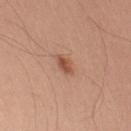A roughly 15 mm field-of-view crop from a total-body skin photograph.
From the arm.
A male subject, in their mid- to late 40s.